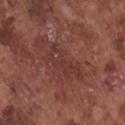Impression:
The lesion was photographed on a routine skin check and not biopsied; there is no pathology result.
Acquisition and patient details:
A region of skin cropped from a whole-body photographic capture, roughly 15 mm wide. Measured at roughly 5.5 mm in maximum diameter. On the chest. The subject is a male aged approximately 75.